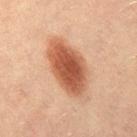follow-up = imaged on a skin check; not biopsied
automated metrics = an area of roughly 21 mm², a shape eccentricity near 0.85, and two-axis asymmetry of about 0.15; an average lesion color of about L≈48 a*≈23 b*≈31 (CIELAB) and a normalized border contrast of about 10
size = about 7.5 mm
body site = the right thigh
acquisition = ~15 mm tile from a whole-body skin photo
patient = female, aged 18 to 22